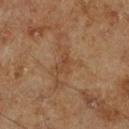Q: Patient demographics?
A: male, aged 68 to 72
Q: What kind of image is this?
A: ~15 mm tile from a whole-body skin photo
Q: What is the anatomic site?
A: the right lower leg